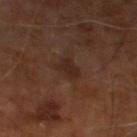biopsy_status: not biopsied; imaged during a skin examination
automated_metrics:
  cielab_L: 24
  cielab_a: 17
  cielab_b: 22
  vs_skin_darker_L: 6.0
  vs_skin_contrast_norm: 7.0
  border_irregularity_0_10: 3.0
  peripheral_color_asymmetry: 0.5
image:
  source: total-body photography crop
  field_of_view_mm: 15
lesion_size:
  long_diameter_mm_approx: 2.5
site: right leg
patient:
  sex: male
  age_approx: 60